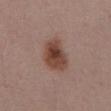Assessment: Imaged during a routine full-body skin examination; the lesion was not biopsied and no histopathology is available.